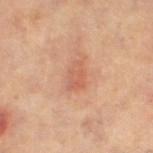diameter = ~3 mm (longest diameter); site = the left thigh; illumination = cross-polarized; subject = female, aged 38–42; imaging modality = total-body-photography crop, ~15 mm field of view.